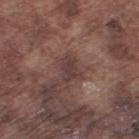This lesion was catalogued during total-body skin photography and was not selected for biopsy. A roughly 15 mm field-of-view crop from a total-body skin photograph. A male subject, aged 73–77. The lesion is located on the right lower leg.